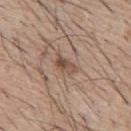Part of a total-body skin-imaging series; this lesion was reviewed on a skin check and was not flagged for biopsy.
On the mid back.
The patient is a male aged around 65.
Cropped from a total-body skin-imaging series; the visible field is about 15 mm.